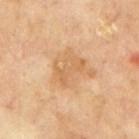lighting: cross-polarized
image:
  source: total-body photography crop
  field_of_view_mm: 15
patient:
  sex: male
  age_approx: 70
site: mid back